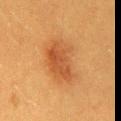Part of a total-body skin-imaging series; this lesion was reviewed on a skin check and was not flagged for biopsy. The patient is a female in their 40s. An algorithmic analysis of the crop reported a mean CIELAB color near L≈44 a*≈23 b*≈36, roughly 8 lightness units darker than nearby skin, and a lesion-to-skin contrast of about 6.5 (normalized; higher = more distinct). This is a cross-polarized tile. Approximately 5.5 mm at its widest. The lesion is located on the lower back. A 15 mm crop from a total-body photograph taken for skin-cancer surveillance.